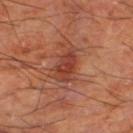Case summary:
* workup · catalogued during a skin exam; not biopsied
* subject · roughly 65 years of age
* body site · the right thigh
* image · ~15 mm tile from a whole-body skin photo